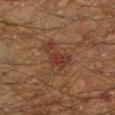site: left lower leg
image:
  source: total-body photography crop
  field_of_view_mm: 15
patient:
  sex: male
  age_approx: 60
lesion_size:
  long_diameter_mm_approx: 4.5
automated_metrics:
  area_mm2_approx: 9.5
  eccentricity: 0.7
  cielab_L: 27
  cielab_a: 18
  cielab_b: 22
  vs_skin_darker_L: 6.0
  vs_skin_contrast_norm: 6.5